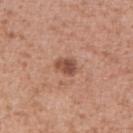Impression: The lesion was photographed on a routine skin check and not biopsied; there is no pathology result. Context: On the arm. Longest diameter approximately 2.5 mm. A 15 mm close-up tile from a total-body photography series done for melanoma screening. A female patient, about 30 years old. Imaged with white-light lighting.